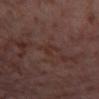Part of a total-body skin-imaging series; this lesion was reviewed on a skin check and was not flagged for biopsy.
The lesion is on the right thigh.
The subject is a female in their mid- to late 50s.
A lesion tile, about 15 mm wide, cut from a 3D total-body photograph.